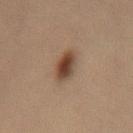Q: Is there a histopathology result?
A: total-body-photography surveillance lesion; no biopsy
Q: Who is the patient?
A: female, roughly 60 years of age
Q: What is the imaging modality?
A: 15 mm crop, total-body photography
Q: What did automated image analysis measure?
A: a lesion area of about 7.5 mm² and a shape-asymmetry score of about 0.15 (0 = symmetric); a nevus-likeness score of about 100/100
Q: What lighting was used for the tile?
A: cross-polarized
Q: What is the anatomic site?
A: the lower back
Q: Lesion size?
A: about 3.5 mm